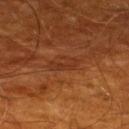notes — no biopsy performed (imaged during a skin exam) | site — the back | imaging modality — 15 mm crop, total-body photography | subject — male, approximately 60 years of age | tile lighting — cross-polarized | automated lesion analysis — an outline eccentricity of about 0.9 (0 = round, 1 = elongated); a mean CIELAB color near L≈31 a*≈24 b*≈31, roughly 5 lightness units darker than nearby skin, and a normalized border contrast of about 5; a classifier nevus-likeness of about 0/100.